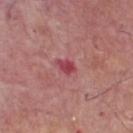Q: Is there a histopathology result?
A: catalogued during a skin exam; not biopsied
Q: What did automated image analysis measure?
A: a lesion area of about 3.5 mm², an eccentricity of roughly 0.8, and a shape-asymmetry score of about 0.2 (0 = symmetric); a mean CIELAB color near L≈46 a*≈36 b*≈20, about 11 CIELAB-L* units darker than the surrounding skin, and a normalized border contrast of about 8; a border-irregularity rating of about 2/10, internal color variation of about 2 on a 0–10 scale, and a peripheral color-asymmetry measure near 0.5; a nevus-likeness score of about 0/100 and lesion-presence confidence of about 100/100
Q: Lesion location?
A: the front of the torso
Q: Patient demographics?
A: male, aged approximately 75
Q: What kind of image is this?
A: total-body-photography crop, ~15 mm field of view
Q: How large is the lesion?
A: ≈2.5 mm
Q: Illumination type?
A: white-light illumination